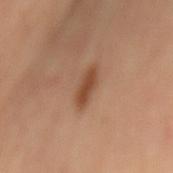This lesion was catalogued during total-body skin photography and was not selected for biopsy. Located on the back. The patient is a female aged around 60. The lesion's longest dimension is about 4.5 mm. Automated image analysis of the tile measured a border-irregularity index near 3/10, a within-lesion color-variation index near 1.5/10, and a peripheral color-asymmetry measure near 0.5. The analysis additionally found a classifier nevus-likeness of about 80/100 and a lesion-detection confidence of about 100/100. Imaged with cross-polarized lighting. A region of skin cropped from a whole-body photographic capture, roughly 15 mm wide.